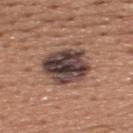Case summary:
– notes · imaged on a skin check; not biopsied
– subject · male, aged 43–47
– image source · ~15 mm crop, total-body skin-cancer survey
– image-analysis metrics · a footprint of about 19 mm², a shape eccentricity near 0.55, and a shape-asymmetry score of about 0.15 (0 = symmetric); roughly 17 lightness units darker than nearby skin; a nevus-likeness score of about 5/100 and lesion-presence confidence of about 100/100
– lesion diameter · about 5.5 mm
– tile lighting · white-light
– anatomic site · the back